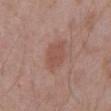{"biopsy_status": "not biopsied; imaged during a skin examination", "automated_metrics": {"cielab_L": 51, "cielab_a": 22, "cielab_b": 25, "vs_skin_darker_L": 7.0, "border_irregularity_0_10": 1.5, "color_variation_0_10": 2.0, "peripheral_color_asymmetry": 0.5, "lesion_detection_confidence_0_100": 100}, "lighting": "white-light", "site": "chest", "lesion_size": {"long_diameter_mm_approx": 4.0}, "patient": {"sex": "male", "age_approx": 70}, "image": {"source": "total-body photography crop", "field_of_view_mm": 15}}